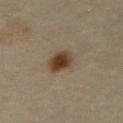<record>
  <biopsy_status>not biopsied; imaged during a skin examination</biopsy_status>
  <patient>
    <sex>female</sex>
    <age_approx>40</age_approx>
  </patient>
  <automated_metrics>
    <border_irregularity_0_10>1.5</border_irregularity_0_10>
    <color_variation_0_10>5.5</color_variation_0_10>
    <peripheral_color_asymmetry>1.5</peripheral_color_asymmetry>
    <nevus_likeness_0_100>100</nevus_likeness_0_100>
  </automated_metrics>
  <lighting>cross-polarized</lighting>
  <image>
    <source>total-body photography crop</source>
    <field_of_view_mm>15</field_of_view_mm>
  </image>
  <lesion_size>
    <long_diameter_mm_approx>3.0</long_diameter_mm_approx>
  </lesion_size>
  <site>right thigh</site>
</record>